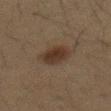Q: Was a biopsy performed?
A: catalogued during a skin exam; not biopsied
Q: Who is the patient?
A: male, aged around 35
Q: What kind of image is this?
A: total-body-photography crop, ~15 mm field of view
Q: Where on the body is the lesion?
A: the abdomen
Q: What lighting was used for the tile?
A: cross-polarized illumination